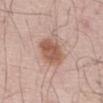biopsy status=imaged on a skin check; not biopsied | imaging modality=~15 mm tile from a whole-body skin photo | illumination=white-light | location=the abdomen | patient=male, approximately 60 years of age | automated lesion analysis=a footprint of about 11 mm² and a shape eccentricity near 0.5; a detector confidence of about 100 out of 100 that the crop contains a lesion.